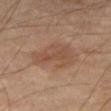  biopsy_status: not biopsied; imaged during a skin examination
  site: right thigh
  image:
    source: total-body photography crop
    field_of_view_mm: 15
  lesion_size:
    long_diameter_mm_approx: 6.0
  patient:
    sex: male
    age_approx: 70
  automated_metrics:
    area_mm2_approx: 16.0
    eccentricity: 0.8
    shape_asymmetry: 0.3
  lighting: cross-polarized